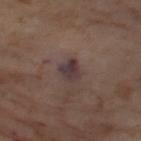Case summary:
- biopsy status — imaged on a skin check; not biopsied
- patient — female, in their mid- to late 50s
- site — the left thigh
- image source — 15 mm crop, total-body photography